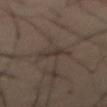Q: Was a biopsy performed?
A: catalogued during a skin exam; not biopsied
Q: How was the tile lit?
A: cross-polarized
Q: What is the imaging modality?
A: 15 mm crop, total-body photography
Q: Lesion location?
A: the front of the torso
Q: Lesion size?
A: about 3 mm
Q: What are the patient's age and sex?
A: male, in their 60s